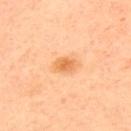{"biopsy_status": "not biopsied; imaged during a skin examination", "automated_metrics": {"shape_asymmetry": 0.25, "nevus_likeness_0_100": 95, "lesion_detection_confidence_0_100": 100}, "lesion_size": {"long_diameter_mm_approx": 3.0}, "lighting": "cross-polarized", "site": "upper back", "image": {"source": "total-body photography crop", "field_of_view_mm": 15}, "patient": {"sex": "male", "age_approx": 40}}